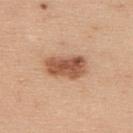biopsy status=catalogued during a skin exam; not biopsied
acquisition=total-body-photography crop, ~15 mm field of view
subject=male, roughly 35 years of age
location=the upper back
diameter=about 5 mm